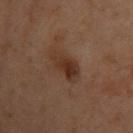Notes:
- notes · imaged on a skin check; not biopsied
- subject · female, roughly 55 years of age
- automated metrics · a footprint of about 7.5 mm², a shape eccentricity near 0.8, and a shape-asymmetry score of about 0.25 (0 = symmetric); a border-irregularity index near 2.5/10, a color-variation rating of about 4.5/10, and radial color variation of about 1.5
- lighting · cross-polarized
- anatomic site · the upper back
- lesion size · ≈4 mm
- image · ~15 mm tile from a whole-body skin photo